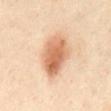follow-up=total-body-photography surveillance lesion; no biopsy | illumination=cross-polarized illumination | patient=male, aged approximately 50 | image=~15 mm tile from a whole-body skin photo | anatomic site=the abdomen.